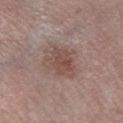A close-up tile cropped from a whole-body skin photograph, about 15 mm across. Located on the right lower leg. Measured at roughly 4 mm in maximum diameter. A male subject, about 55 years old. The tile uses white-light illumination.